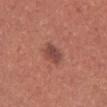Case summary:
– workup: catalogued during a skin exam; not biopsied
– lighting: white-light illumination
– image source: 15 mm crop, total-body photography
– lesion size: ≈3 mm
– body site: the upper back
– patient: female, aged approximately 35